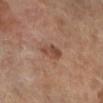workup: total-body-photography surveillance lesion; no biopsy
patient: female, aged 68 to 72
diameter: ≈3.5 mm
body site: the leg
image source: total-body-photography crop, ~15 mm field of view
lighting: cross-polarized illumination